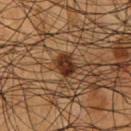- follow-up — no biopsy performed (imaged during a skin exam)
- acquisition — ~15 mm tile from a whole-body skin photo
- location — the chest
- size — ~3 mm (longest diameter)
- subject — male, approximately 55 years of age